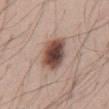| key | value |
|---|---|
| follow-up | total-body-photography surveillance lesion; no biopsy |
| patient | male, aged approximately 45 |
| anatomic site | the abdomen |
| lesion size | about 4.5 mm |
| image-analysis metrics | an outline eccentricity of about 0.7 (0 = round, 1 = elongated); a border-irregularity index near 1.5/10, a within-lesion color-variation index near 7.5/10, and peripheral color asymmetry of about 2; an automated nevus-likeness rating near 95 out of 100 and a lesion-detection confidence of about 100/100 |
| tile lighting | white-light |
| image source | total-body-photography crop, ~15 mm field of view |